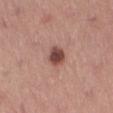This image is a 15 mm lesion crop taken from a total-body photograph.
From the left thigh.
The tile uses white-light illumination.
Longest diameter approximately 3 mm.
A male subject aged 73 to 77.
Automated tile analysis of the lesion measured a border-irregularity index near 2/10, internal color variation of about 3.5 on a 0–10 scale, and radial color variation of about 1. The analysis additionally found lesion-presence confidence of about 100/100.